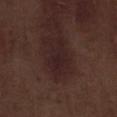Captured during whole-body skin photography for melanoma surveillance; the lesion was not biopsied.
A lesion tile, about 15 mm wide, cut from a 3D total-body photograph.
The patient is a male aged 68–72.
From the right lower leg.
An algorithmic analysis of the crop reported a lesion area of about 7 mm², an outline eccentricity of about 0.85 (0 = round, 1 = elongated), and a shape-asymmetry score of about 0.35 (0 = symmetric). And it measured a mean CIELAB color near L≈21 a*≈16 b*≈14 and roughly 6 lightness units darker than nearby skin. The analysis additionally found a border-irregularity index near 4/10, internal color variation of about 1.5 on a 0–10 scale, and a peripheral color-asymmetry measure near 0.5. The analysis additionally found a lesion-detection confidence of about 100/100.
Captured under white-light illumination.